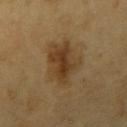Case summary:
* biopsy status: total-body-photography surveillance lesion; no biopsy
* imaging modality: ~15 mm tile from a whole-body skin photo
* site: the arm
* subject: male, aged 58 to 62